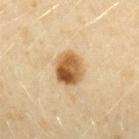image source — total-body-photography crop, ~15 mm field of view | TBP lesion metrics — an area of roughly 9.5 mm², an outline eccentricity of about 0.55 (0 = round, 1 = elongated), and a symmetry-axis asymmetry near 0.15; a color-variation rating of about 9.5/10 | size — about 4 mm | body site — the arm | tile lighting — cross-polarized | patient — female, aged approximately 40.